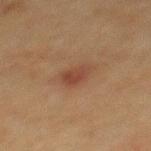No biopsy was performed on this lesion — it was imaged during a full skin examination and was not determined to be concerning. A male patient in their 50s. The tile uses cross-polarized illumination. From the mid back. A 15 mm close-up extracted from a 3D total-body photography capture. Approximately 3 mm at its widest.